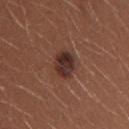| field | value |
|---|---|
| notes | no biopsy performed (imaged during a skin exam) |
| image | total-body-photography crop, ~15 mm field of view |
| subject | female, aged 23 to 27 |
| diameter | about 3 mm |
| lighting | white-light |
| location | the arm |